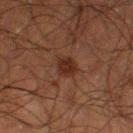{
  "biopsy_status": "not biopsied; imaged during a skin examination",
  "lesion_size": {
    "long_diameter_mm_approx": 2.5
  },
  "lighting": "cross-polarized",
  "patient": {
    "sex": "male",
    "age_approx": 50
  },
  "image": {
    "source": "total-body photography crop",
    "field_of_view_mm": 15
  },
  "site": "left thigh"
}